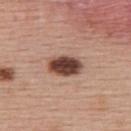workup: total-body-photography surveillance lesion; no biopsy | subject: female, in their mid- to late 50s | diameter: ≈4 mm | site: the upper back | acquisition: 15 mm crop, total-body photography | automated lesion analysis: an area of roughly 9 mm², an outline eccentricity of about 0.8 (0 = round, 1 = elongated), and a symmetry-axis asymmetry near 0.1; a within-lesion color-variation index near 5/10 and a peripheral color-asymmetry measure near 1.5 | lighting: white-light illumination.